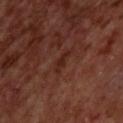Findings:
– imaging modality — 15 mm crop, total-body photography
– automated metrics — an area of roughly 3 mm² and an outline eccentricity of about 0.9 (0 = round, 1 = elongated); a lesion–skin lightness drop of about 5; an automated nevus-likeness rating near 0 out of 100 and a detector confidence of about 95 out of 100 that the crop contains a lesion
– illumination — cross-polarized illumination
– anatomic site — the back
– patient — male, aged approximately 70